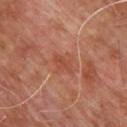The lesion was photographed on a routine skin check and not biopsied; there is no pathology result. The patient is a male in their mid-60s. Imaged with cross-polarized lighting. From the upper back. This image is a 15 mm lesion crop taken from a total-body photograph.